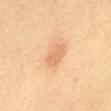Context:
The lesion's longest dimension is about 3.5 mm. The patient is a female in their mid- to late 50s. A lesion tile, about 15 mm wide, cut from a 3D total-body photograph. From the chest.